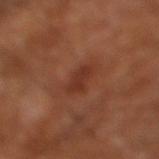Findings:
• notes: catalogued during a skin exam; not biopsied
• automated lesion analysis: a mean CIELAB color near L≈33 a*≈25 b*≈29 and about 7 CIELAB-L* units darker than the surrounding skin; an automated nevus-likeness rating near 0 out of 100
• diameter: ~3 mm (longest diameter)
• illumination: cross-polarized illumination
• acquisition: ~15 mm crop, total-body skin-cancer survey
• site: the leg
• subject: aged approximately 65